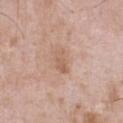notes = no biopsy performed (imaged during a skin exam)
lighting = white-light illumination
acquisition = ~15 mm tile from a whole-body skin photo
patient = male, aged 48 to 52
location = the front of the torso
lesion diameter = about 3 mm